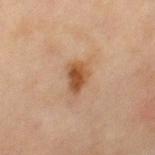workup: catalogued during a skin exam; not biopsied
image: 15 mm crop, total-body photography
illumination: cross-polarized illumination
subject: female, aged around 70
automated metrics: an automated nevus-likeness rating near 90 out of 100 and lesion-presence confidence of about 100/100
lesion diameter: ≈3.5 mm
body site: the right thigh